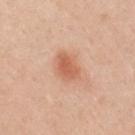Part of a total-body skin-imaging series; this lesion was reviewed on a skin check and was not flagged for biopsy. A 15 mm close-up tile from a total-body photography series done for melanoma screening. An algorithmic analysis of the crop reported an average lesion color of about L≈61 a*≈26 b*≈34 (CIELAB) and a lesion-to-skin contrast of about 7 (normalized; higher = more distinct). It also reported a nevus-likeness score of about 95/100 and a detector confidence of about 100 out of 100 that the crop contains a lesion. A male patient, approximately 50 years of age. On the arm. This is a white-light tile. The recorded lesion diameter is about 3.5 mm.